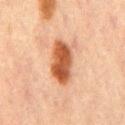Q: Is there a histopathology result?
A: imaged on a skin check; not biopsied
Q: Lesion size?
A: ≈5 mm
Q: What lighting was used for the tile?
A: cross-polarized
Q: Automated lesion metrics?
A: a mean CIELAB color near L≈44 a*≈23 b*≈32, a lesion–skin lightness drop of about 15, and a lesion-to-skin contrast of about 11.5 (normalized; higher = more distinct)
Q: Where on the body is the lesion?
A: the mid back
Q: What are the patient's age and sex?
A: male, aged approximately 65
Q: What kind of image is this?
A: 15 mm crop, total-body photography